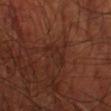Impression: The lesion was photographed on a routine skin check and not biopsied; there is no pathology result. Clinical summary: A male patient aged 68–72. Measured at roughly 4 mm in maximum diameter. A lesion tile, about 15 mm wide, cut from a 3D total-body photograph. Located on the left lower leg. The total-body-photography lesion software estimated an area of roughly 4.5 mm², an outline eccentricity of about 0.95 (0 = round, 1 = elongated), and two-axis asymmetry of about 0.4. The analysis additionally found an average lesion color of about L≈24 a*≈20 b*≈23 (CIELAB) and roughly 4 lightness units darker than nearby skin. Imaged with cross-polarized lighting.